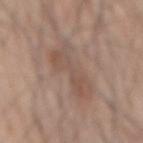This lesion was catalogued during total-body skin photography and was not selected for biopsy. Longest diameter approximately 7 mm. Cropped from a total-body skin-imaging series; the visible field is about 15 mm. A male subject, roughly 70 years of age. From the mid back. This is a white-light tile.